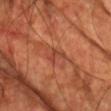site: the chest
subject: male, aged 58–62
diameter: about 3 mm
tile lighting: cross-polarized
automated lesion analysis: a lesion area of about 4 mm², an eccentricity of roughly 0.8, and a symmetry-axis asymmetry near 0.45; border irregularity of about 4.5 on a 0–10 scale and internal color variation of about 2.5 on a 0–10 scale; a detector confidence of about 85 out of 100 that the crop contains a lesion
acquisition: total-body-photography crop, ~15 mm field of view